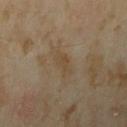location: the right upper arm
illumination: cross-polarized
patient: female, about 35 years old
lesion diameter: about 3.5 mm
imaging modality: ~15 mm tile from a whole-body skin photo